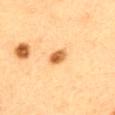Q: Was a biopsy performed?
A: total-body-photography surveillance lesion; no biopsy
Q: What kind of image is this?
A: ~15 mm tile from a whole-body skin photo
Q: What are the patient's age and sex?
A: female, roughly 30 years of age
Q: What lighting was used for the tile?
A: cross-polarized
Q: How large is the lesion?
A: ≈2.5 mm
Q: Lesion location?
A: the upper back